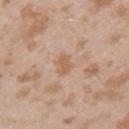Part of a total-body skin-imaging series; this lesion was reviewed on a skin check and was not flagged for biopsy.
The lesion is located on the left upper arm.
The patient is a female roughly 25 years of age.
About 2.5 mm across.
Captured under white-light illumination.
An algorithmic analysis of the crop reported a mean CIELAB color near L≈60 a*≈17 b*≈32 and a normalized lesion–skin contrast near 6. It also reported a border-irregularity rating of about 2.5/10 and peripheral color asymmetry of about 0.5. It also reported an automated nevus-likeness rating near 0 out of 100 and a lesion-detection confidence of about 100/100.
A 15 mm close-up tile from a total-body photography series done for melanoma screening.